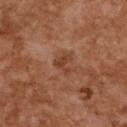Cropped from a total-body skin-imaging series; the visible field is about 15 mm. Longest diameter approximately 2.5 mm. The lesion is located on the upper back. The subject is a female about 60 years old.